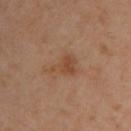The lesion was tiled from a total-body skin photograph and was not biopsied. On the right upper arm. A female patient in their 60s. Captured under cross-polarized illumination. Approximately 3.5 mm at its widest. A 15 mm close-up extracted from a 3D total-body photography capture.